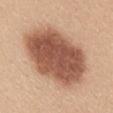Case summary:
* subject: female, roughly 30 years of age
* acquisition: ~15 mm crop, total-body skin-cancer survey
* site: the mid back
* tile lighting: white-light illumination